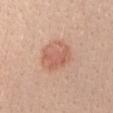biopsy_status: not biopsied; imaged during a skin examination
lighting: white-light
patient:
  sex: female
  age_approx: 25
automated_metrics:
  cielab_L: 61
  cielab_a: 23
  cielab_b: 30
  vs_skin_darker_L: 9.0
  vs_skin_contrast_norm: 6.0
  color_variation_0_10: 4.0
  peripheral_color_asymmetry: 1.5
  lesion_detection_confidence_0_100: 100
site: left forearm
image:
  source: total-body photography crop
  field_of_view_mm: 15
lesion_size:
  long_diameter_mm_approx: 4.5